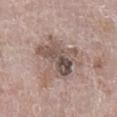No biopsy was performed on this lesion — it was imaged during a full skin examination and was not determined to be concerning.
The recorded lesion diameter is about 6.5 mm.
Cropped from a whole-body photographic skin survey; the tile spans about 15 mm.
The lesion is located on the right lower leg.
The patient is a male roughly 65 years of age.
This is a white-light tile.